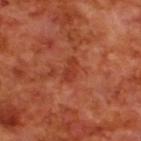Notes:
– workup — total-body-photography surveillance lesion; no biopsy
– site — the back
– patient — male, aged approximately 70
– imaging modality — 15 mm crop, total-body photography